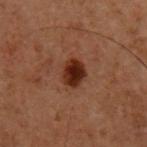No biopsy was performed on this lesion — it was imaged during a full skin examination and was not determined to be concerning.
A lesion tile, about 15 mm wide, cut from a 3D total-body photograph.
The lesion is located on the chest.
Automated tile analysis of the lesion measured an area of roughly 6.5 mm² and a symmetry-axis asymmetry near 0.2. The software also gave a mean CIELAB color near L≈20 a*≈19 b*≈22, roughly 11 lightness units darker than nearby skin, and a normalized lesion–skin contrast near 13. And it measured border irregularity of about 2 on a 0–10 scale and internal color variation of about 4 on a 0–10 scale.
A male patient aged 58–62.